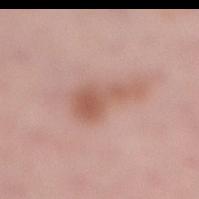biopsy status: no biopsy performed (imaged during a skin exam) | subject: female, in their mid- to late 60s | body site: the right lower leg | tile lighting: white-light | lesion diameter: about 6 mm | image: 15 mm crop, total-body photography | TBP lesion metrics: an eccentricity of roughly 0.9 and a shape-asymmetry score of about 0.55 (0 = symmetric); an average lesion color of about L≈57 a*≈22 b*≈28 (CIELAB), a lesion–skin lightness drop of about 10, and a normalized lesion–skin contrast near 7.5.